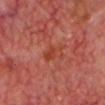biopsy_status: not biopsied; imaged during a skin examination
lesion_size:
  long_diameter_mm_approx: 3.0
site: head or neck
patient:
  sex: male
  age_approx: 65
lighting: cross-polarized
image:
  source: total-body photography crop
  field_of_view_mm: 15